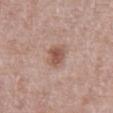On the abdomen.
A lesion tile, about 15 mm wide, cut from a 3D total-body photograph.
About 3 mm across.
Imaged with white-light lighting.
A male patient, aged 68 to 72.